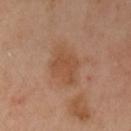A female patient about 40 years old.
A 15 mm close-up tile from a total-body photography series done for melanoma screening.
Located on the left upper arm.